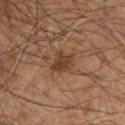Findings:
– notes — catalogued during a skin exam; not biopsied
– subject — male, in their 60s
– body site — the chest
– illumination — cross-polarized illumination
– diameter — ~3 mm (longest diameter)
– image — 15 mm crop, total-body photography